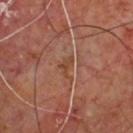notes: total-body-photography surveillance lesion; no biopsy | patient: male, in their 60s | lesion diameter: about 3 mm | lighting: cross-polarized illumination | anatomic site: the upper back | image: ~15 mm crop, total-body skin-cancer survey | automated lesion analysis: an average lesion color of about L≈42 a*≈22 b*≈30 (CIELAB), about 6 CIELAB-L* units darker than the surrounding skin, and a lesion-to-skin contrast of about 6 (normalized; higher = more distinct).